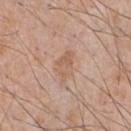Q: Was this lesion biopsied?
A: total-body-photography surveillance lesion; no biopsy
Q: Lesion location?
A: the front of the torso
Q: Who is the patient?
A: male, aged 53–57
Q: What is the imaging modality?
A: ~15 mm tile from a whole-body skin photo